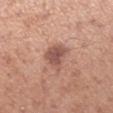Findings:
• follow-up: total-body-photography surveillance lesion; no biopsy
• body site: the left forearm
• image: ~15 mm crop, total-body skin-cancer survey
• patient: female, aged 38 to 42